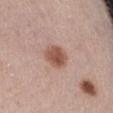site: the abdomen
image source: total-body-photography crop, ~15 mm field of view
automated lesion analysis: two-axis asymmetry of about 0.15; border irregularity of about 1.5 on a 0–10 scale, a color-variation rating of about 3.5/10, and peripheral color asymmetry of about 1
tile lighting: white-light illumination
patient: female, aged 63 to 67